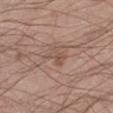{"biopsy_status": "not biopsied; imaged during a skin examination", "site": "leg", "image": {"source": "total-body photography crop", "field_of_view_mm": 15}, "patient": {"sex": "male", "age_approx": 40}, "lesion_size": {"long_diameter_mm_approx": 3.0}}